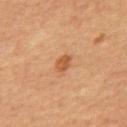Notes:
– follow-up — imaged on a skin check; not biopsied
– patient — male, aged approximately 65
– location — the chest
– imaging modality — ~15 mm tile from a whole-body skin photo